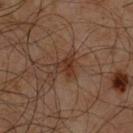Q: Automated lesion metrics?
A: an average lesion color of about L≈30 a*≈17 b*≈25 (CIELAB), roughly 7 lightness units darker than nearby skin, and a normalized lesion–skin contrast near 7; a within-lesion color-variation index near 3.5/10 and a peripheral color-asymmetry measure near 1; a nevus-likeness score of about 10/100
Q: What is the lesion's diameter?
A: about 2.5 mm
Q: Patient demographics?
A: male, roughly 60 years of age
Q: What kind of image is this?
A: 15 mm crop, total-body photography
Q: Lesion location?
A: the chest
Q: How was the tile lit?
A: cross-polarized